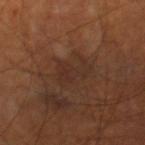biopsy status: catalogued during a skin exam; not biopsied
anatomic site: the right thigh
lighting: cross-polarized
patient: male, aged 68–72
automated metrics: an area of roughly 7.5 mm² and a shape eccentricity near 0.75; a classifier nevus-likeness of about 0/100 and a detector confidence of about 80 out of 100 that the crop contains a lesion
imaging modality: ~15 mm crop, total-body skin-cancer survey